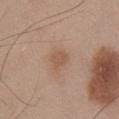Imaged during a routine full-body skin examination; the lesion was not biopsied and no histopathology is available. A close-up tile cropped from a whole-body skin photograph, about 15 mm across. From the chest. The subject is a male approximately 55 years of age. Captured under white-light illumination.